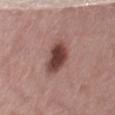biopsy_status: not biopsied; imaged during a skin examination
automated_metrics:
  shape_asymmetry: 0.25
  cielab_L: 43
  cielab_a: 21
  cielab_b: 23
  vs_skin_darker_L: 15.0
  vs_skin_contrast_norm: 11.0
image:
  source: total-body photography crop
  field_of_view_mm: 15
patient:
  sex: female
  age_approx: 75
site: right thigh